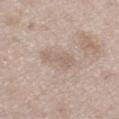follow-up: catalogued during a skin exam; not biopsied
image source: ~15 mm tile from a whole-body skin photo
location: the left thigh
patient: male, aged around 50
lesion diameter: ~4 mm (longest diameter)
illumination: white-light